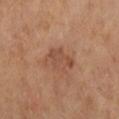Impression: Recorded during total-body skin imaging; not selected for excision or biopsy. Context: A female patient, in their mid-60s. Approximately 4 mm at its widest. Imaged with cross-polarized lighting. An algorithmic analysis of the crop reported a mean CIELAB color near L≈48 a*≈21 b*≈30, a lesion–skin lightness drop of about 8, and a normalized border contrast of about 5.5. The lesion is located on the arm. A 15 mm close-up tile from a total-body photography series done for melanoma screening.